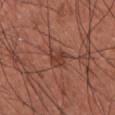Clinical impression: The lesion was tiled from a total-body skin photograph and was not biopsied. Context: From the chest. The recorded lesion diameter is about 3 mm. The subject is a male aged 33–37. Cropped from a whole-body photographic skin survey; the tile spans about 15 mm. This is a white-light tile.